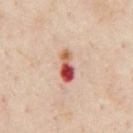<tbp_lesion>
<biopsy_status>not biopsied; imaged during a skin examination</biopsy_status>
<patient>
  <sex>male</sex>
  <age_approx>55</age_approx>
</patient>
<image>
  <source>total-body photography crop</source>
  <field_of_view_mm>15</field_of_view_mm>
</image>
<lighting>cross-polarized</lighting>
<automated_metrics>
  <border_irregularity_0_10>2.5</border_irregularity_0_10>
  <color_variation_0_10>10.0</color_variation_0_10>
</automated_metrics>
<site>chest</site>
</tbp_lesion>